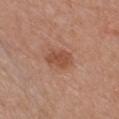Clinical impression: Part of a total-body skin-imaging series; this lesion was reviewed on a skin check and was not flagged for biopsy. Image and clinical context: A female subject in their 50s. From the front of the torso. A 15 mm crop from a total-body photograph taken for skin-cancer surveillance. The lesion's longest dimension is about 4 mm. Imaged with white-light lighting.